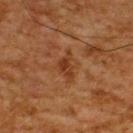Imaged during a routine full-body skin examination; the lesion was not biopsied and no histopathology is available.
This is a cross-polarized tile.
A male subject, roughly 60 years of age.
Cropped from a whole-body photographic skin survey; the tile spans about 15 mm.
Located on the upper back.
The lesion's longest dimension is about 3.5 mm.